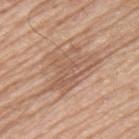notes = imaged on a skin check; not biopsied | imaging modality = total-body-photography crop, ~15 mm field of view | patient = male, aged approximately 75 | anatomic site = the upper back | tile lighting = white-light illumination.